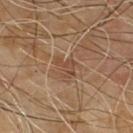Assessment: The lesion was tiled from a total-body skin photograph and was not biopsied. Image and clinical context: Captured under cross-polarized illumination. The total-body-photography lesion software estimated a lesion area of about 3.5 mm², an outline eccentricity of about 0.8 (0 = round, 1 = elongated), and a symmetry-axis asymmetry near 0.65. The software also gave an average lesion color of about L≈44 a*≈19 b*≈29 (CIELAB), about 7 CIELAB-L* units darker than the surrounding skin, and a normalized border contrast of about 6. And it measured a classifier nevus-likeness of about 0/100. Approximately 3 mm at its widest. A male patient aged 63 to 67. This image is a 15 mm lesion crop taken from a total-body photograph.